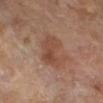Measured at roughly 4.5 mm in maximum diameter.
Cropped from a whole-body photographic skin survey; the tile spans about 15 mm.
On the leg.
A female patient about 70 years old.